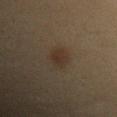image source: 15 mm crop, total-body photography
image-analysis metrics: a border-irregularity index near 2.5/10, internal color variation of about 2.5 on a 0–10 scale, and peripheral color asymmetry of about 1; an automated nevus-likeness rating near 75 out of 100 and a detector confidence of about 100 out of 100 that the crop contains a lesion
subject: female, aged 28 to 32
lesion diameter: about 3.5 mm
body site: the left forearm